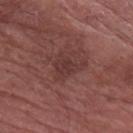Part of a total-body skin-imaging series; this lesion was reviewed on a skin check and was not flagged for biopsy.